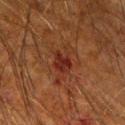| key | value |
|---|---|
| workup | total-body-photography surveillance lesion; no biopsy |
| site | the right upper arm |
| image | ~15 mm crop, total-body skin-cancer survey |
| lighting | cross-polarized illumination |
| patient | male, in their mid-60s |